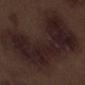A male subject, aged approximately 70. Located on the left thigh. A lesion tile, about 15 mm wide, cut from a 3D total-body photograph.